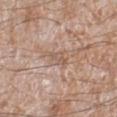Case summary:
• follow-up: catalogued during a skin exam; not biopsied
• image source: 15 mm crop, total-body photography
• diameter: about 2.5 mm
• lighting: white-light
• anatomic site: the left lower leg
• patient: male, roughly 60 years of age